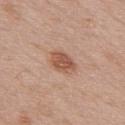Imaged during a routine full-body skin examination; the lesion was not biopsied and no histopathology is available. Captured under white-light illumination. A male subject aged around 70. From the upper back. Cropped from a total-body skin-imaging series; the visible field is about 15 mm. The lesion's longest dimension is about 3.5 mm.